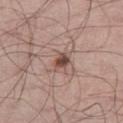Impression:
Imaged during a routine full-body skin examination; the lesion was not biopsied and no histopathology is available.
Image and clinical context:
A 15 mm close-up extracted from a 3D total-body photography capture. Measured at roughly 2.5 mm in maximum diameter. From the right thigh. The tile uses white-light illumination. A male subject in their mid-50s.